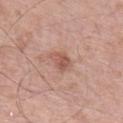- notes: catalogued during a skin exam; not biopsied
- tile lighting: white-light illumination
- size: about 3 mm
- body site: the leg
- subject: male, aged 78 to 82
- imaging modality: 15 mm crop, total-body photography
- image-analysis metrics: a border-irregularity rating of about 3/10, internal color variation of about 4 on a 0–10 scale, and a peripheral color-asymmetry measure near 1.5